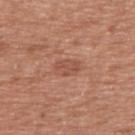Impression: The lesion was photographed on a routine skin check and not biopsied; there is no pathology result. Acquisition and patient details: A 15 mm close-up tile from a total-body photography series done for melanoma screening. The lesion is on the upper back. A male patient, aged 73 to 77.